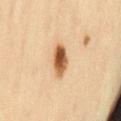Captured during whole-body skin photography for melanoma surveillance; the lesion was not biopsied. A female subject roughly 45 years of age. The tile uses cross-polarized illumination. A region of skin cropped from a whole-body photographic capture, roughly 15 mm wide. On the lower back.